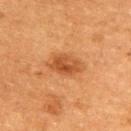biopsy_status: not biopsied; imaged during a skin examination